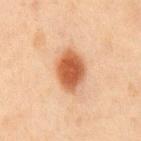Clinical impression:
The lesion was photographed on a routine skin check and not biopsied; there is no pathology result.
Context:
A male patient, about 45 years old. A lesion tile, about 15 mm wide, cut from a 3D total-body photograph. On the chest. About 5 mm across.